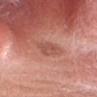The lesion was tiled from a total-body skin photograph and was not biopsied.
A roughly 15 mm field-of-view crop from a total-body skin photograph.
The lesion is on the head or neck.
The tile uses white-light illumination.
A male patient, about 45 years old.
The lesion-visualizer software estimated a footprint of about 6.5 mm² and an outline eccentricity of about 0.75 (0 = round, 1 = elongated). It also reported an average lesion color of about L≈54 a*≈26 b*≈29 (CIELAB), a lesion–skin lightness drop of about 8, and a normalized lesion–skin contrast near 6. And it measured a nevus-likeness score of about 0/100 and lesion-presence confidence of about 100/100.